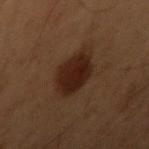No biopsy was performed on this lesion — it was imaged during a full skin examination and was not determined to be concerning. A male subject, aged 53–57. A 15 mm close-up extracted from a 3D total-body photography capture. The lesion's longest dimension is about 4 mm. This is a cross-polarized tile. The lesion-visualizer software estimated roughly 8 lightness units darker than nearby skin and a normalized lesion–skin contrast near 11. The analysis additionally found border irregularity of about 1.5 on a 0–10 scale, internal color variation of about 2.5 on a 0–10 scale, and peripheral color asymmetry of about 0.5. On the right upper arm.